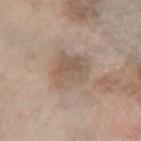workup: total-body-photography surveillance lesion; no biopsy
subject: female, aged approximately 70
image source: total-body-photography crop, ~15 mm field of view
anatomic site: the arm